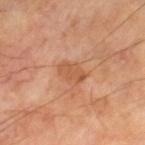Approximately 3.5 mm at its widest.
Cropped from a whole-body photographic skin survey; the tile spans about 15 mm.
The lesion is located on the leg.
A male subject aged approximately 70.